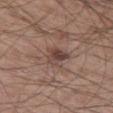follow-up: no biopsy performed (imaged during a skin exam) | location: the left thigh | tile lighting: white-light | subject: male, aged 58 to 62 | image-analysis metrics: a lesion area of about 5.5 mm², an outline eccentricity of about 0.25 (0 = round, 1 = elongated), and a symmetry-axis asymmetry near 0.35; a lesion color around L≈43 a*≈17 b*≈22 in CIELAB, a lesion–skin lightness drop of about 10, and a normalized border contrast of about 8 | imaging modality: 15 mm crop, total-body photography.